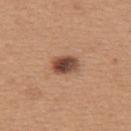No biopsy was performed on this lesion — it was imaged during a full skin examination and was not determined to be concerning. Measured at roughly 3 mm in maximum diameter. A female subject aged 28–32. The tile uses white-light illumination. A close-up tile cropped from a whole-body skin photograph, about 15 mm across. From the upper back.